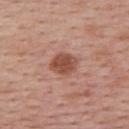biopsy status: catalogued during a skin exam; not biopsied | patient: female, in their mid- to late 50s | imaging modality: ~15 mm tile from a whole-body skin photo | automated metrics: an outline eccentricity of about 0.55 (0 = round, 1 = elongated) and a shape-asymmetry score of about 0.15 (0 = symmetric); an average lesion color of about L≈50 a*≈24 b*≈29 (CIELAB), a lesion–skin lightness drop of about 12, and a normalized lesion–skin contrast near 9; a color-variation rating of about 2.5/10 and peripheral color asymmetry of about 1; a classifier nevus-likeness of about 95/100 and a detector confidence of about 100 out of 100 that the crop contains a lesion | site: the upper back | illumination: white-light illumination.